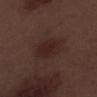notes — no biopsy performed (imaged during a skin exam); patient — male, about 70 years old; size — ≈4 mm; tile lighting — white-light illumination; image — 15 mm crop, total-body photography; body site — the left lower leg.